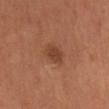follow-up — total-body-photography surveillance lesion; no biopsy | body site — the mid back | subject — male, approximately 55 years of age | image — total-body-photography crop, ~15 mm field of view | automated metrics — a mean CIELAB color near L≈37 a*≈21 b*≈29 and a lesion–skin lightness drop of about 8; border irregularity of about 2.5 on a 0–10 scale, a color-variation rating of about 2/10, and peripheral color asymmetry of about 0.5 | lesion diameter — ≈2.5 mm.